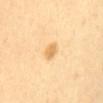Part of a total-body skin-imaging series; this lesion was reviewed on a skin check and was not flagged for biopsy.
Measured at roughly 2.5 mm in maximum diameter.
The lesion is located on the front of the torso.
Automated tile analysis of the lesion measured a footprint of about 3.5 mm², a shape eccentricity near 0.7, and two-axis asymmetry of about 0.2. And it measured a lesion color around L≈75 a*≈18 b*≈47 in CIELAB and roughly 10 lightness units darker than nearby skin. The analysis additionally found a nevus-likeness score of about 80/100.
The patient is a female aged 53–57.
A region of skin cropped from a whole-body photographic capture, roughly 15 mm wide.
This is a cross-polarized tile.